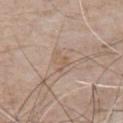• follow-up: total-body-photography surveillance lesion; no biopsy
• subject: male, roughly 80 years of age
• image-analysis metrics: a footprint of about 3 mm²; a border-irregularity index near 7/10, a within-lesion color-variation index near 0/10, and a peripheral color-asymmetry measure near 0; a classifier nevus-likeness of about 0/100
• tile lighting: white-light illumination
• anatomic site: the chest
• acquisition: total-body-photography crop, ~15 mm field of view
• size: ≈2.5 mm